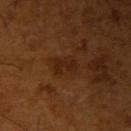<case>
<biopsy_status>not biopsied; imaged during a skin examination</biopsy_status>
<patient>
  <sex>male</sex>
  <age_approx>65</age_approx>
</patient>
<site>right upper arm</site>
<image>
  <source>total-body photography crop</source>
  <field_of_view_mm>15</field_of_view_mm>
</image>
</case>